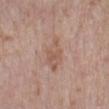{"biopsy_status": "not biopsied; imaged during a skin examination"}